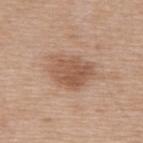The lesion was photographed on a routine skin check and not biopsied; there is no pathology result. The lesion is on the upper back. A male patient aged around 70. Cropped from a whole-body photographic skin survey; the tile spans about 15 mm. Imaged with white-light lighting. An algorithmic analysis of the crop reported a footprint of about 15 mm², an eccentricity of roughly 0.7, and a shape-asymmetry score of about 0.2 (0 = symmetric). And it measured a lesion color around L≈55 a*≈19 b*≈31 in CIELAB and a lesion–skin lightness drop of about 10. The analysis additionally found a within-lesion color-variation index near 3.5/10 and a peripheral color-asymmetry measure near 1.5. And it measured an automated nevus-likeness rating near 20 out of 100 and a lesion-detection confidence of about 100/100.